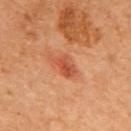The lesion was tiled from a total-body skin photograph and was not biopsied.
The subject is a female approximately 65 years of age.
Cropped from a total-body skin-imaging series; the visible field is about 15 mm.
The total-body-photography lesion software estimated an area of roughly 5 mm², a shape eccentricity near 0.85, and a symmetry-axis asymmetry near 0.4. And it measured about 10 CIELAB-L* units darker than the surrounding skin. The software also gave border irregularity of about 3.5 on a 0–10 scale and radial color variation of about 1.5. And it measured a classifier nevus-likeness of about 95/100 and a detector confidence of about 100 out of 100 that the crop contains a lesion.
About 3.5 mm across.
The lesion is located on the upper back.